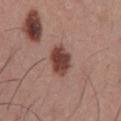Part of a total-body skin-imaging series; this lesion was reviewed on a skin check and was not flagged for biopsy. Approximately 4 mm at its widest. The lesion is on the front of the torso. This is a white-light tile. The patient is a male aged 38–42. A 15 mm close-up tile from a total-body photography series done for melanoma screening. Automated image analysis of the tile measured a classifier nevus-likeness of about 75/100 and lesion-presence confidence of about 100/100.